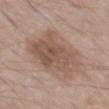Q: Was a biopsy performed?
A: catalogued during a skin exam; not biopsied
Q: What lighting was used for the tile?
A: white-light
Q: Where on the body is the lesion?
A: the left thigh
Q: What are the patient's age and sex?
A: male, in their mid- to late 60s
Q: How was this image acquired?
A: total-body-photography crop, ~15 mm field of view
Q: How large is the lesion?
A: ~7.5 mm (longest diameter)